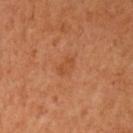This lesion was catalogued during total-body skin photography and was not selected for biopsy.
The recorded lesion diameter is about 2.5 mm.
On the left upper arm.
A female subject, approximately 55 years of age.
A lesion tile, about 15 mm wide, cut from a 3D total-body photograph.
Captured under cross-polarized illumination.